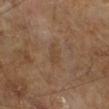Q: Was a biopsy performed?
A: imaged on a skin check; not biopsied
Q: How was this image acquired?
A: 15 mm crop, total-body photography
Q: What are the patient's age and sex?
A: male, approximately 65 years of age
Q: What lighting was used for the tile?
A: cross-polarized illumination